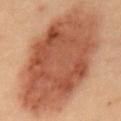Assessment: Part of a total-body skin-imaging series; this lesion was reviewed on a skin check and was not flagged for biopsy.